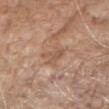{"biopsy_status": "not biopsied; imaged during a skin examination", "site": "arm", "patient": {"sex": "female", "age_approx": "85+"}, "lighting": "white-light", "lesion_size": {"long_diameter_mm_approx": 3.0}, "automated_metrics": {"eccentricity": 0.9, "shape_asymmetry": 0.65, "border_irregularity_0_10": 7.5, "color_variation_0_10": 0.0, "peripheral_color_asymmetry": 0.0, "nevus_likeness_0_100": 0, "lesion_detection_confidence_0_100": 95}, "image": {"source": "total-body photography crop", "field_of_view_mm": 15}}